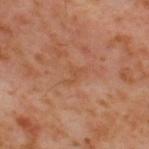follow-up=catalogued during a skin exam; not biopsied | lesion size=~2.5 mm (longest diameter) | acquisition=15 mm crop, total-body photography | site=the upper back | illumination=cross-polarized illumination | subject=male, aged 58 to 62.